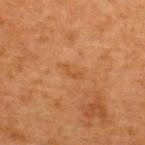The lesion was tiled from a total-body skin photograph and was not biopsied.
The lesion is located on the upper back.
A close-up tile cropped from a whole-body skin photograph, about 15 mm across.
A male subject, roughly 65 years of age.
The lesion's longest dimension is about 3 mm.